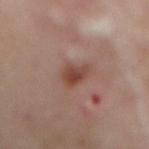The subject is a female approximately 60 years of age.
The lesion is located on the mid back.
Measured at roughly 3 mm in maximum diameter.
The tile uses cross-polarized illumination.
The lesion-visualizer software estimated a lesion area of about 5 mm² and a shape eccentricity near 0.6.
Cropped from a whole-body photographic skin survey; the tile spans about 15 mm.